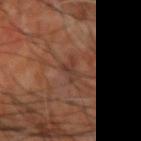An algorithmic analysis of the crop reported a footprint of about 3.5 mm², a shape eccentricity near 0.75, and two-axis asymmetry of about 0.5. The software also gave a lesion color around L≈36 a*≈20 b*≈25 in CIELAB and a normalized lesion–skin contrast near 5.5. Measured at roughly 2.5 mm in maximum diameter. The subject is in their mid-60s. A roughly 15 mm field-of-view crop from a total-body skin photograph. Captured under cross-polarized illumination. Located on the right upper arm.